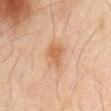Q: Is there a histopathology result?
A: total-body-photography surveillance lesion; no biopsy
Q: Who is the patient?
A: male, aged approximately 65
Q: Automated lesion metrics?
A: an eccentricity of roughly 0.7 and a shape-asymmetry score of about 0.3 (0 = symmetric); an average lesion color of about L≈59 a*≈20 b*≈35 (CIELAB), roughly 8 lightness units darker than nearby skin, and a lesion-to-skin contrast of about 6.5 (normalized; higher = more distinct); internal color variation of about 3 on a 0–10 scale and radial color variation of about 1; a classifier nevus-likeness of about 35/100 and a detector confidence of about 100 out of 100 that the crop contains a lesion
Q: What kind of image is this?
A: 15 mm crop, total-body photography
Q: Where on the body is the lesion?
A: the mid back
Q: What lighting was used for the tile?
A: cross-polarized
Q: How large is the lesion?
A: ~3.5 mm (longest diameter)